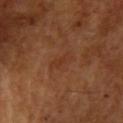biopsy_status: not biopsied; imaged during a skin examination
site: chest
automated_metrics:
  vs_skin_darker_L: 5.0
  vs_skin_contrast_norm: 4.5
  border_irregularity_0_10: 3.0
  color_variation_0_10: 0.0
lesion_size:
  long_diameter_mm_approx: 2.5
image:
  source: total-body photography crop
  field_of_view_mm: 15
patient:
  sex: male
  age_approx: 65
lighting: cross-polarized